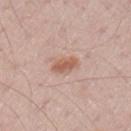Findings:
- follow-up · imaged on a skin check; not biopsied
- subject · male, in their 50s
- site · the left upper arm
- acquisition · ~15 mm crop, total-body skin-cancer survey
- size · ~3 mm (longest diameter)
- illumination · white-light illumination